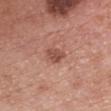patient: female, about 60 years old | illumination: white-light | acquisition: total-body-photography crop, ~15 mm field of view | body site: the front of the torso.